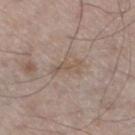Q: Was a biopsy performed?
A: total-body-photography surveillance lesion; no biopsy
Q: Patient demographics?
A: male, aged approximately 60
Q: Lesion size?
A: ≈4 mm
Q: How was the tile lit?
A: white-light
Q: What is the anatomic site?
A: the left lower leg
Q: What did automated image analysis measure?
A: a classifier nevus-likeness of about 0/100 and a detector confidence of about 90 out of 100 that the crop contains a lesion
Q: What is the imaging modality?
A: ~15 mm crop, total-body skin-cancer survey